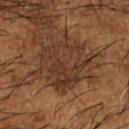Q: Was this lesion biopsied?
A: catalogued during a skin exam; not biopsied
Q: What kind of image is this?
A: 15 mm crop, total-body photography
Q: Where on the body is the lesion?
A: the left forearm
Q: Lesion size?
A: ≈6 mm
Q: What are the patient's age and sex?
A: male, aged around 65
Q: What did automated image analysis measure?
A: a footprint of about 21 mm²; a lesion color around L≈35 a*≈19 b*≈28 in CIELAB, roughly 6 lightness units darker than nearby skin, and a normalized lesion–skin contrast near 6.5; a border-irregularity rating of about 6.5/10, a color-variation rating of about 4.5/10, and a peripheral color-asymmetry measure near 1.5
Q: What lighting was used for the tile?
A: cross-polarized